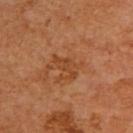Impression: The lesion was photographed on a routine skin check and not biopsied; there is no pathology result. Image and clinical context: The lesion is located on the upper back. This image is a 15 mm lesion crop taken from a total-body photograph. Measured at roughly 3.5 mm in maximum diameter. The patient is a male roughly 60 years of age.